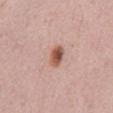workup: total-body-photography surveillance lesion; no biopsy
lighting: white-light
patient: male, approximately 60 years of age
lesion diameter: about 2.5 mm
site: the abdomen
image: ~15 mm crop, total-body skin-cancer survey